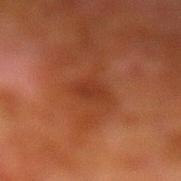Q: Automated lesion metrics?
A: a lesion color around L≈28 a*≈23 b*≈28 in CIELAB, a lesion–skin lightness drop of about 5, and a normalized border contrast of about 5.5; a border-irregularity index near 1.5/10, a within-lesion color-variation index near 1.5/10, and peripheral color asymmetry of about 0.5; an automated nevus-likeness rating near 0 out of 100
Q: What is the anatomic site?
A: the left lower leg
Q: What is the lesion's diameter?
A: ~2.5 mm (longest diameter)
Q: What kind of image is this?
A: ~15 mm tile from a whole-body skin photo
Q: Patient demographics?
A: male, aged approximately 80
Q: What lighting was used for the tile?
A: cross-polarized